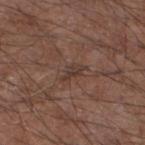Assessment:
Part of a total-body skin-imaging series; this lesion was reviewed on a skin check and was not flagged for biopsy.
Background:
The patient is a male approximately 55 years of age. The recorded lesion diameter is about 2.5 mm. Cropped from a total-body skin-imaging series; the visible field is about 15 mm. The lesion is located on the left lower leg.